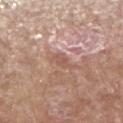Captured during whole-body skin photography for melanoma surveillance; the lesion was not biopsied.
The lesion-visualizer software estimated a mean CIELAB color near L≈55 a*≈20 b*≈27 and a lesion-to-skin contrast of about 5.5 (normalized; higher = more distinct). And it measured a nevus-likeness score of about 0/100 and lesion-presence confidence of about 80/100.
This image is a 15 mm lesion crop taken from a total-body photograph.
The patient is a male aged approximately 65.
From the arm.
Measured at roughly 3 mm in maximum diameter.